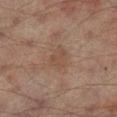Part of a total-body skin-imaging series; this lesion was reviewed on a skin check and was not flagged for biopsy. On the right lower leg. A roughly 15 mm field-of-view crop from a total-body skin photograph. A male subject approximately 65 years of age. Imaged with cross-polarized lighting.